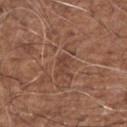Recorded during total-body skin imaging; not selected for excision or biopsy. Captured under white-light illumination. A male patient aged 73 to 77. About 2.5 mm across. Located on the right upper arm. A 15 mm close-up tile from a total-body photography series done for melanoma screening.